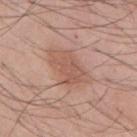Impression:
This lesion was catalogued during total-body skin photography and was not selected for biopsy.
Clinical summary:
The tile uses white-light illumination. The lesion is on the chest. The subject is a male in their mid- to late 50s. A close-up tile cropped from a whole-body skin photograph, about 15 mm across. An algorithmic analysis of the crop reported a footprint of about 13 mm², an eccentricity of roughly 0.8, and two-axis asymmetry of about 0.3. And it measured an average lesion color of about L≈56 a*≈21 b*≈28 (CIELAB), a lesion–skin lightness drop of about 8, and a normalized border contrast of about 5.5. The software also gave a border-irregularity rating of about 3.5/10, internal color variation of about 2.5 on a 0–10 scale, and peripheral color asymmetry of about 1. The analysis additionally found an automated nevus-likeness rating near 35 out of 100. Approximately 5.5 mm at its widest.